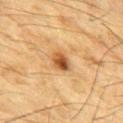biopsy status: imaged on a skin check; not biopsied | image: total-body-photography crop, ~15 mm field of view | automated metrics: a footprint of about 4.5 mm² and an eccentricity of roughly 0.65; a border-irregularity index near 2.5/10 and peripheral color asymmetry of about 2.5; an automated nevus-likeness rating near 85 out of 100 and lesion-presence confidence of about 100/100 | body site: the chest | lighting: cross-polarized | patient: male, in their mid- to late 80s or older.